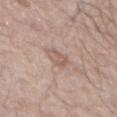Q: Was this lesion biopsied?
A: total-body-photography surveillance lesion; no biopsy
Q: Automated lesion metrics?
A: a shape eccentricity near 0.7 and a symmetry-axis asymmetry near 0.3; a mean CIELAB color near L≈58 a*≈17 b*≈24, about 8 CIELAB-L* units darker than the surrounding skin, and a normalized border contrast of about 5
Q: What is the imaging modality?
A: 15 mm crop, total-body photography
Q: Who is the patient?
A: male, aged 68 to 72
Q: Where on the body is the lesion?
A: the chest
Q: Lesion size?
A: ≈3 mm